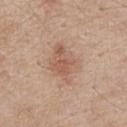{"biopsy_status": "not biopsied; imaged during a skin examination", "patient": {"sex": "male", "age_approx": 65}, "image": {"source": "total-body photography crop", "field_of_view_mm": 15}, "lesion_size": {"long_diameter_mm_approx": 5.0}, "site": "chest", "lighting": "white-light"}